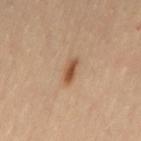Q: Was this lesion biopsied?
A: catalogued during a skin exam; not biopsied
Q: How large is the lesion?
A: about 3.5 mm
Q: What did automated image analysis measure?
A: a footprint of about 4 mm² and an eccentricity of roughly 0.9; a border-irregularity index near 2.5/10, internal color variation of about 4 on a 0–10 scale, and radial color variation of about 1; an automated nevus-likeness rating near 95 out of 100 and a lesion-detection confidence of about 100/100
Q: What is the anatomic site?
A: the right leg
Q: Who is the patient?
A: female, aged 38–42
Q: Illumination type?
A: cross-polarized
Q: How was this image acquired?
A: ~15 mm tile from a whole-body skin photo